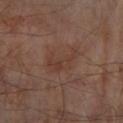This lesion was catalogued during total-body skin photography and was not selected for biopsy. The total-body-photography lesion software estimated a border-irregularity rating of about 4.5/10, a within-lesion color-variation index near 3/10, and peripheral color asymmetry of about 1. The lesion is on the right forearm. Captured under cross-polarized illumination. Longest diameter approximately 4 mm. Cropped from a whole-body photographic skin survey; the tile spans about 15 mm. The patient is a male about 65 years old.